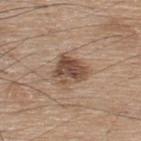<record>
  <biopsy_status>not biopsied; imaged during a skin examination</biopsy_status>
  <patient>
    <sex>male</sex>
    <age_approx>75</age_approx>
  </patient>
  <lighting>white-light</lighting>
  <image>
    <source>total-body photography crop</source>
    <field_of_view_mm>15</field_of_view_mm>
  </image>
  <lesion_size>
    <long_diameter_mm_approx>4.0</long_diameter_mm_approx>
  </lesion_size>
  <automated_metrics>
    <border_irregularity_0_10>3.0</border_irregularity_0_10>
    <color_variation_0_10>5.0</color_variation_0_10>
    <peripheral_color_asymmetry>2.0</peripheral_color_asymmetry>
    <nevus_likeness_0_100>55</nevus_likeness_0_100>
    <lesion_detection_confidence_0_100>100</lesion_detection_confidence_0_100>
  </automated_metrics>
  <site>upper back</site>
</record>